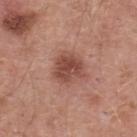- follow-up — no biopsy performed (imaged during a skin exam)